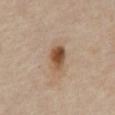Findings:
* notes — total-body-photography surveillance lesion; no biopsy
* patient — male, in their mid- to late 60s
* location — the front of the torso
* automated lesion analysis — about 14 CIELAB-L* units darker than the surrounding skin and a normalized border contrast of about 10.5
* tile lighting — cross-polarized
* lesion diameter — about 3.5 mm
* image source — ~15 mm crop, total-body skin-cancer survey